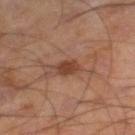No biopsy was performed on this lesion — it was imaged during a full skin examination and was not determined to be concerning.
A male patient in their mid-50s.
Automated tile analysis of the lesion measured a lesion area of about 4 mm², a shape eccentricity near 0.6, and a symmetry-axis asymmetry near 0.15. The analysis additionally found a mean CIELAB color near L≈39 a*≈21 b*≈28 and roughly 9 lightness units darker than nearby skin.
Cropped from a whole-body photographic skin survey; the tile spans about 15 mm.
About 2.5 mm across.
On the right lower leg.